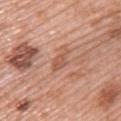Impression:
No biopsy was performed on this lesion — it was imaged during a full skin examination and was not determined to be concerning.
Background:
The lesion is on the back. This is a white-light tile. Measured at roughly 3.5 mm in maximum diameter. A 15 mm close-up tile from a total-body photography series done for melanoma screening. Automated image analysis of the tile measured a footprint of about 4.5 mm², a shape eccentricity near 0.85, and two-axis asymmetry of about 0.4. The analysis additionally found border irregularity of about 4 on a 0–10 scale, a color-variation rating of about 1.5/10, and peripheral color asymmetry of about 0.5. A female patient, approximately 60 years of age.